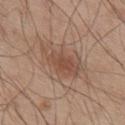{"patient": {"sex": "male", "age_approx": 55}, "image": {"source": "total-body photography crop", "field_of_view_mm": 15}, "site": "back"}